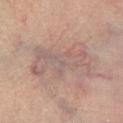No biopsy was performed on this lesion — it was imaged during a full skin examination and was not determined to be concerning. The subject is a female in their 30s. This image is a 15 mm lesion crop taken from a total-body photograph. On the right lower leg. Imaged with cross-polarized lighting. Measured at roughly 7.5 mm in maximum diameter. The total-body-photography lesion software estimated a border-irregularity rating of about 6.5/10, a color-variation rating of about 5/10, and a peripheral color-asymmetry measure near 1.5. And it measured a nevus-likeness score of about 0/100 and lesion-presence confidence of about 55/100.